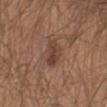{
  "biopsy_status": "not biopsied; imaged during a skin examination",
  "lighting": "white-light",
  "image": {
    "source": "total-body photography crop",
    "field_of_view_mm": 15
  },
  "lesion_size": {
    "long_diameter_mm_approx": 3.5
  },
  "patient": {
    "sex": "male",
    "age_approx": 65
  },
  "site": "abdomen"
}